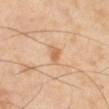The lesion was photographed on a routine skin check and not biopsied; there is no pathology result. The tile uses cross-polarized illumination. From the right lower leg. An algorithmic analysis of the crop reported an automated nevus-likeness rating near 50 out of 100. A roughly 15 mm field-of-view crop from a total-body skin photograph. About 2.5 mm across. A female subject, aged approximately 70.